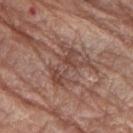| field | value |
|---|---|
| biopsy status | catalogued during a skin exam; not biopsied |
| subject | female, about 75 years old |
| image-analysis metrics | a mean CIELAB color near L≈45 a*≈20 b*≈25, a lesion–skin lightness drop of about 8, and a lesion-to-skin contrast of about 6.5 (normalized; higher = more distinct); a border-irregularity index near 8.5/10, a within-lesion color-variation index near 5.5/10, and radial color variation of about 1.5; a nevus-likeness score of about 0/100 and a detector confidence of about 85 out of 100 that the crop contains a lesion |
| anatomic site | the left forearm |
| illumination | white-light illumination |
| imaging modality | ~15 mm tile from a whole-body skin photo |
| lesion size | ≈5 mm |